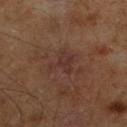Findings:
- workup · total-body-photography surveillance lesion; no biopsy
- lesion diameter · ~3 mm (longest diameter)
- tile lighting · cross-polarized
- patient · male, aged 58 to 62
- site · the left lower leg
- imaging modality · ~15 mm crop, total-body skin-cancer survey
- image-analysis metrics · a lesion area of about 5.5 mm², an outline eccentricity of about 0.4 (0 = round, 1 = elongated), and two-axis asymmetry of about 0.6; a mean CIELAB color near L≈29 a*≈17 b*≈20, a lesion–skin lightness drop of about 5, and a lesion-to-skin contrast of about 5.5 (normalized; higher = more distinct); a border-irregularity rating of about 6.5/10 and radial color variation of about 0.5